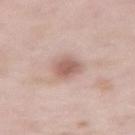<case>
<biopsy_status>not biopsied; imaged during a skin examination</biopsy_status>
<site>abdomen</site>
<image>
  <source>total-body photography crop</source>
  <field_of_view_mm>15</field_of_view_mm>
</image>
<patient>
  <sex>male</sex>
  <age_approx>55</age_approx>
</patient>
<lighting>white-light</lighting>
</case>